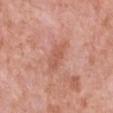notes: total-body-photography surveillance lesion; no biopsy | location: the front of the torso | size: ~3.5 mm (longest diameter) | subject: female, aged 48 to 52 | imaging modality: total-body-photography crop, ~15 mm field of view | tile lighting: white-light.